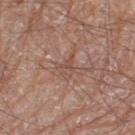follow-up = catalogued during a skin exam; not biopsied
lesion size = about 3 mm
subject = male, aged 78 to 82
tile lighting = white-light
image = 15 mm crop, total-body photography
image-analysis metrics = a mean CIELAB color near L≈50 a*≈20 b*≈26 and about 6 CIELAB-L* units darker than the surrounding skin
site = the left leg